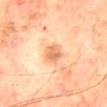Assessment:
Part of a total-body skin-imaging series; this lesion was reviewed on a skin check and was not flagged for biopsy.
Acquisition and patient details:
The lesion is located on the mid back. Automated image analysis of the tile measured a lesion area of about 5.5 mm², an eccentricity of roughly 0.8, and a shape-asymmetry score of about 0.15 (0 = symmetric). The software also gave a border-irregularity rating of about 2/10, a color-variation rating of about 3/10, and peripheral color asymmetry of about 1. The software also gave a classifier nevus-likeness of about 15/100. A roughly 15 mm field-of-view crop from a total-body skin photograph. This is a cross-polarized tile. The patient is a male in their mid-70s. About 3.5 mm across.